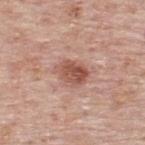Assessment: Captured during whole-body skin photography for melanoma surveillance; the lesion was not biopsied.